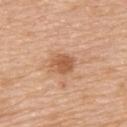Imaged during a routine full-body skin examination; the lesion was not biopsied and no histopathology is available. A female patient aged 63 to 67. A 15 mm close-up tile from a total-body photography series done for melanoma screening. About 2.5 mm across. Located on the back.